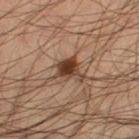biopsy_status: not biopsied; imaged during a skin examination